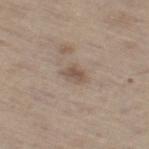notes — no biopsy performed (imaged during a skin exam)
body site — the right thigh
lighting — white-light
TBP lesion metrics — a color-variation rating of about 2.5/10 and radial color variation of about 1
subject — male, aged 68 to 72
image source — ~15 mm crop, total-body skin-cancer survey